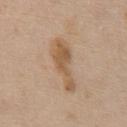Clinical impression: This lesion was catalogued during total-body skin photography and was not selected for biopsy. Clinical summary: Automated image analysis of the tile measured a shape eccentricity near 0.95. And it measured a mean CIELAB color near L≈57 a*≈16 b*≈34, roughly 9 lightness units darker than nearby skin, and a normalized border contrast of about 7.5. And it measured border irregularity of about 5 on a 0–10 scale, a color-variation rating of about 3/10, and a peripheral color-asymmetry measure near 1. The analysis additionally found a classifier nevus-likeness of about 10/100. The tile uses white-light illumination. The lesion is on the chest. The subject is a female about 70 years old. Approximately 6.5 mm at its widest. Cropped from a total-body skin-imaging series; the visible field is about 15 mm.